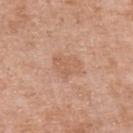notes: imaged on a skin check; not biopsied | image source: total-body-photography crop, ~15 mm field of view | illumination: white-light illumination | automated metrics: an eccentricity of roughly 0.55 and two-axis asymmetry of about 0.3; a lesion color around L≈60 a*≈21 b*≈32 in CIELAB, roughly 6 lightness units darker than nearby skin, and a normalized border contrast of about 4.5; a border-irregularity index near 3/10, internal color variation of about 2 on a 0–10 scale, and radial color variation of about 0.5 | location: the left forearm | subject: female, about 60 years old | lesion size: ~3.5 mm (longest diameter).